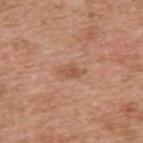Assessment:
The lesion was tiled from a total-body skin photograph and was not biopsied.
Image and clinical context:
The lesion-visualizer software estimated a shape eccentricity near 0.85 and two-axis asymmetry of about 0.25. The software also gave a lesion–skin lightness drop of about 8 and a lesion-to-skin contrast of about 6 (normalized; higher = more distinct). The subject is a male aged 58 to 62. On the back. Cropped from a total-body skin-imaging series; the visible field is about 15 mm. About 3 mm across. This is a white-light tile.